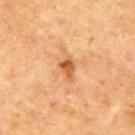biopsy status: imaged on a skin check; not biopsied
site: the upper back
lighting: cross-polarized
patient: male, aged approximately 70
image source: ~15 mm tile from a whole-body skin photo
lesion size: ~2.5 mm (longest diameter)
automated lesion analysis: a mean CIELAB color near L≈47 a*≈22 b*≈36, about 11 CIELAB-L* units darker than the surrounding skin, and a normalized border contrast of about 8; a classifier nevus-likeness of about 5/100